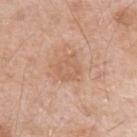Cropped from a whole-body photographic skin survey; the tile spans about 15 mm. Longest diameter approximately 3.5 mm. The subject is a male aged around 55. The lesion is located on the left upper arm.